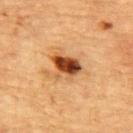biopsy status: total-body-photography surveillance lesion; no biopsy
image source: total-body-photography crop, ~15 mm field of view
patient: female, aged 68 to 72
image-analysis metrics: a lesion area of about 9 mm², an outline eccentricity of about 0.6 (0 = round, 1 = elongated), and a shape-asymmetry score of about 0.35 (0 = symmetric); an average lesion color of about L≈41 a*≈22 b*≈35 (CIELAB) and a lesion-to-skin contrast of about 12 (normalized; higher = more distinct); a lesion-detection confidence of about 100/100
anatomic site: the upper back